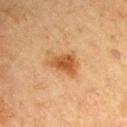biopsy status: no biopsy performed (imaged during a skin exam)
patient: male, roughly 65 years of age
image source: total-body-photography crop, ~15 mm field of view
location: the left upper arm